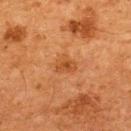follow-up — no biopsy performed (imaged during a skin exam) | patient — male, about 65 years old | body site — the upper back | acquisition — total-body-photography crop, ~15 mm field of view.